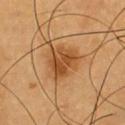Context: Automated image analysis of the tile measured an area of roughly 13 mm², an outline eccentricity of about 0.5 (0 = round, 1 = elongated), and two-axis asymmetry of about 0.3. The software also gave a lesion color around L≈50 a*≈23 b*≈41 in CIELAB and about 11 CIELAB-L* units darker than the surrounding skin. Located on the chest. A 15 mm close-up extracted from a 3D total-body photography capture. A male subject, roughly 60 years of age. Measured at roughly 4.5 mm in maximum diameter.